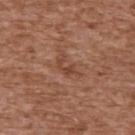| field | value |
|---|---|
| biopsy status | no biopsy performed (imaged during a skin exam) |
| image source | 15 mm crop, total-body photography |
| subject | male, in their mid-70s |
| site | the upper back |